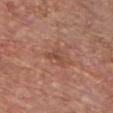| feature | finding |
|---|---|
| follow-up | no biopsy performed (imaged during a skin exam) |
| site | the front of the torso |
| diameter | ~2.5 mm (longest diameter) |
| TBP lesion metrics | a border-irregularity index near 6.5/10, a color-variation rating of about 1/10, and radial color variation of about 0 |
| acquisition | ~15 mm tile from a whole-body skin photo |
| tile lighting | white-light |
| patient | male, in their mid- to late 60s |